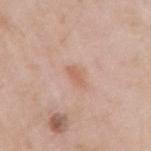Assessment: This lesion was catalogued during total-body skin photography and was not selected for biopsy.